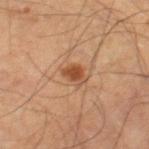No biopsy was performed on this lesion — it was imaged during a full skin examination and was not determined to be concerning. A male subject, in their 60s. Approximately 2.5 mm at its widest. The lesion is located on the left thigh. A roughly 15 mm field-of-view crop from a total-body skin photograph. This is a cross-polarized tile.